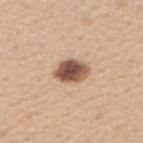follow-up: catalogued during a skin exam; not biopsied
subject: female, aged approximately 40
imaging modality: ~15 mm crop, total-body skin-cancer survey
anatomic site: the arm